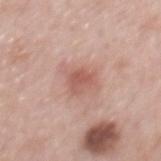  lesion_size:
    long_diameter_mm_approx: 2.5
  image:
    source: total-body photography crop
    field_of_view_mm: 15
  patient:
    sex: male
    age_approx: 65
  lighting: white-light
  site: mid back